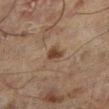biopsy status: imaged on a skin check; not biopsied
patient: male, about 60 years old
automated lesion analysis: a footprint of about 3.5 mm², an eccentricity of roughly 0.75, and a shape-asymmetry score of about 0.25 (0 = symmetric); a lesion color around L≈31 a*≈14 b*≈24 in CIELAB and a normalized lesion–skin contrast near 9; a border-irregularity index near 2/10, a within-lesion color-variation index near 2/10, and radial color variation of about 0.5
lighting: cross-polarized illumination
imaging modality: 15 mm crop, total-body photography
size: ~2.5 mm (longest diameter)
anatomic site: the left lower leg